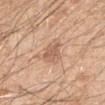workup — catalogued during a skin exam; not biopsied
tile lighting — white-light illumination
image — ~15 mm tile from a whole-body skin photo
location — the left upper arm
subject — male, approximately 50 years of age